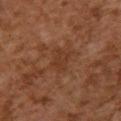Clinical impression:
The lesion was photographed on a routine skin check and not biopsied; there is no pathology result.
Context:
Cropped from a total-body skin-imaging series; the visible field is about 15 mm. The subject is a female about 60 years old. The lesion is located on the back. The tile uses cross-polarized illumination. The recorded lesion diameter is about 3 mm.